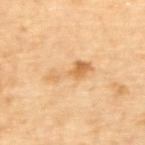Clinical impression:
The lesion was photographed on a routine skin check and not biopsied; there is no pathology result.
Clinical summary:
About 5.5 mm across. The patient is a female approximately 65 years of age. An algorithmic analysis of the crop reported an area of roughly 7 mm², an eccentricity of roughly 0.95, and two-axis asymmetry of about 0.5. And it measured an average lesion color of about L≈68 a*≈20 b*≈44 (CIELAB), a lesion–skin lightness drop of about 10, and a lesion-to-skin contrast of about 6.5 (normalized; higher = more distinct). The analysis additionally found border irregularity of about 6.5 on a 0–10 scale and a within-lesion color-variation index near 4/10. And it measured a nevus-likeness score of about 0/100. A 15 mm close-up extracted from a 3D total-body photography capture. Imaged with cross-polarized lighting. On the back.